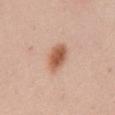Findings:
- notes · no biopsy performed (imaged during a skin exam)
- location · the mid back
- patient · female, aged 38 to 42
- automated lesion analysis · an area of roughly 7.5 mm² and an outline eccentricity of about 0.85 (0 = round, 1 = elongated); border irregularity of about 2 on a 0–10 scale and radial color variation of about 1
- acquisition · 15 mm crop, total-body photography
- lighting · white-light